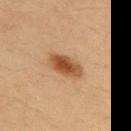Impression: This lesion was catalogued during total-body skin photography and was not selected for biopsy. Image and clinical context: A female subject, aged around 35. Located on the right upper arm. Automated tile analysis of the lesion measured an area of roughly 9.5 mm² and an eccentricity of roughly 0.85. The software also gave a mean CIELAB color near L≈53 a*≈23 b*≈37, about 14 CIELAB-L* units darker than the surrounding skin, and a normalized lesion–skin contrast near 9.5. And it measured an automated nevus-likeness rating near 100 out of 100 and lesion-presence confidence of about 100/100. This image is a 15 mm lesion crop taken from a total-body photograph. Imaged with cross-polarized lighting. About 5 mm across.